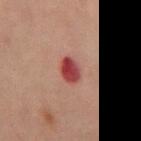Recorded during total-body skin imaging; not selected for excision or biopsy.
A 15 mm close-up extracted from a 3D total-body photography capture.
The subject is a male aged around 70.
Imaged with cross-polarized lighting.
The recorded lesion diameter is about 3 mm.
From the abdomen.